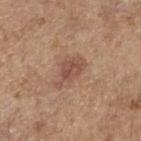Impression: No biopsy was performed on this lesion — it was imaged during a full skin examination and was not determined to be concerning. Clinical summary: A female patient, aged around 65. Captured under white-light illumination. Cropped from a total-body skin-imaging series; the visible field is about 15 mm. From the right upper arm.